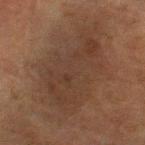Captured during whole-body skin photography for melanoma surveillance; the lesion was not biopsied. A male patient, in their mid-70s. Automated tile analysis of the lesion measured a mean CIELAB color near L≈30 a*≈14 b*≈22. And it measured a border-irregularity index near 5.5/10, internal color variation of about 3 on a 0–10 scale, and radial color variation of about 1. Cropped from a total-body skin-imaging series; the visible field is about 15 mm. From the left forearm. Imaged with cross-polarized lighting.